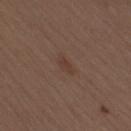The lesion was tiled from a total-body skin photograph and was not biopsied.
A female patient, approximately 40 years of age.
This is a white-light tile.
Located on the leg.
The recorded lesion diameter is about 2.5 mm.
This image is a 15 mm lesion crop taken from a total-body photograph.